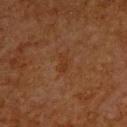biopsy status = no biopsy performed (imaged during a skin exam) | lesion size = ~2.5 mm (longest diameter) | TBP lesion metrics = about 4 CIELAB-L* units darker than the surrounding skin; a peripheral color-asymmetry measure near 0.5; a nevus-likeness score of about 5/100 and lesion-presence confidence of about 100/100 | body site = the back | image source = 15 mm crop, total-body photography | patient = male, aged around 65.